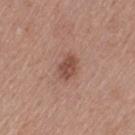Captured during whole-body skin photography for melanoma surveillance; the lesion was not biopsied.
Cropped from a whole-body photographic skin survey; the tile spans about 15 mm.
A female subject approximately 55 years of age.
From the left thigh.
The lesion's longest dimension is about 2.5 mm.
The tile uses white-light illumination.